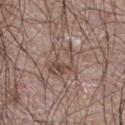Part of a total-body skin-imaging series; this lesion was reviewed on a skin check and was not flagged for biopsy.